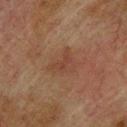| feature | finding |
|---|---|
| follow-up | total-body-photography surveillance lesion; no biopsy |
| lesion size | ~3.5 mm (longest diameter) |
| imaging modality | ~15 mm crop, total-body skin-cancer survey |
| illumination | cross-polarized illumination |
| body site | the upper back |
| subject | male, roughly 75 years of age |